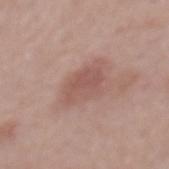Assessment:
Imaged during a routine full-body skin examination; the lesion was not biopsied and no histopathology is available.
Image and clinical context:
A male patient, approximately 60 years of age. The recorded lesion diameter is about 4 mm. This is a white-light tile. From the mid back. The lesion-visualizer software estimated a lesion color around L≈53 a*≈21 b*≈24 in CIELAB, about 8 CIELAB-L* units darker than the surrounding skin, and a normalized border contrast of about 5.5. The software also gave border irregularity of about 3 on a 0–10 scale, internal color variation of about 2 on a 0–10 scale, and peripheral color asymmetry of about 0.5. A 15 mm close-up extracted from a 3D total-body photography capture.